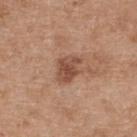follow-up: total-body-photography surveillance lesion; no biopsy
anatomic site: the upper back
subject: female, in their 40s
acquisition: ~15 mm tile from a whole-body skin photo